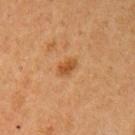{"biopsy_status": "not biopsied; imaged during a skin examination", "automated_metrics": {"area_mm2_approx": 4.0, "eccentricity": 0.75, "shape_asymmetry": 0.25, "nevus_likeness_0_100": 85, "lesion_detection_confidence_0_100": 100}, "patient": {"sex": "female", "age_approx": 60}, "image": {"source": "total-body photography crop", "field_of_view_mm": 15}, "lighting": "cross-polarized", "site": "left upper arm"}